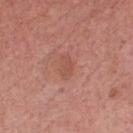Case summary:
* notes — no biopsy performed (imaged during a skin exam)
* body site — the chest
* illumination — white-light
* subject — male, aged 63 to 67
* acquisition — ~15 mm tile from a whole-body skin photo
* lesion size — about 3 mm